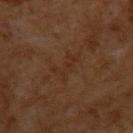- follow-up — imaged on a skin check; not biopsied
- anatomic site — the back
- size — ~3.5 mm (longest diameter)
- lighting — cross-polarized illumination
- imaging modality — ~15 mm crop, total-body skin-cancer survey
- subject — male, aged approximately 60